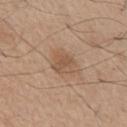{
  "biopsy_status": "not biopsied; imaged during a skin examination",
  "image": {
    "source": "total-body photography crop",
    "field_of_view_mm": 15
  },
  "lighting": "white-light",
  "lesion_size": {
    "long_diameter_mm_approx": 2.5
  },
  "site": "chest",
  "patient": {
    "sex": "male",
    "age_approx": 55
  }
}